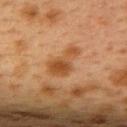Q: Is there a histopathology result?
A: no biopsy performed (imaged during a skin exam)
Q: Illumination type?
A: cross-polarized
Q: What are the patient's age and sex?
A: female, in their 40s
Q: Where on the body is the lesion?
A: the upper back
Q: What kind of image is this?
A: 15 mm crop, total-body photography